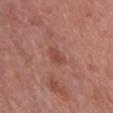* follow-up — imaged on a skin check; not biopsied
* lesion diameter — ≈3 mm
* site — the chest
* tile lighting — white-light illumination
* image — ~15 mm crop, total-body skin-cancer survey
* image-analysis metrics — a color-variation rating of about 1.5/10 and a peripheral color-asymmetry measure near 0.5
* subject — female, aged 58 to 62